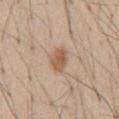Image and clinical context: The patient is a male aged 53–57. The recorded lesion diameter is about 3 mm. This image is a 15 mm lesion crop taken from a total-body photograph. The lesion is on the abdomen.